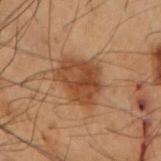Part of a total-body skin-imaging series; this lesion was reviewed on a skin check and was not flagged for biopsy.
Automated image analysis of the tile measured two-axis asymmetry of about 0.25. And it measured an average lesion color of about L≈37 a*≈19 b*≈29 (CIELAB), about 9 CIELAB-L* units darker than the surrounding skin, and a normalized border contrast of about 8. And it measured border irregularity of about 2.5 on a 0–10 scale, internal color variation of about 4 on a 0–10 scale, and radial color variation of about 1.5.
A 15 mm close-up extracted from a 3D total-body photography capture.
The lesion's longest dimension is about 5 mm.
A male subject, roughly 55 years of age.
The lesion is on the left forearm.